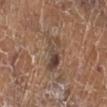| field | value |
|---|---|
| notes | imaged on a skin check; not biopsied |
| subject | female, aged approximately 75 |
| image source | total-body-photography crop, ~15 mm field of view |
| lesion diameter | about 4.5 mm |
| location | the leg |
| lighting | white-light illumination |